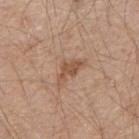biopsy status: imaged on a skin check; not biopsied | lighting: white-light | anatomic site: the right upper arm | patient: male, approximately 60 years of age | automated lesion analysis: a footprint of about 6 mm², an outline eccentricity of about 0.85 (0 = round, 1 = elongated), and a shape-asymmetry score of about 0.45 (0 = symmetric); a lesion color around L≈53 a*≈19 b*≈31 in CIELAB, about 9 CIELAB-L* units darker than the surrounding skin, and a normalized border contrast of about 6.5; a border-irregularity rating of about 5.5/10, internal color variation of about 1 on a 0–10 scale, and a peripheral color-asymmetry measure near 0.5; a classifier nevus-likeness of about 5/100 and a detector confidence of about 100 out of 100 that the crop contains a lesion | lesion diameter: ~4 mm (longest diameter) | image: 15 mm crop, total-body photography.